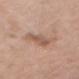This lesion was catalogued during total-body skin photography and was not selected for biopsy. Located on the front of the torso. A female subject roughly 40 years of age. A lesion tile, about 15 mm wide, cut from a 3D total-body photograph. Measured at roughly 3 mm in maximum diameter. Automated tile analysis of the lesion measured a footprint of about 5 mm² and two-axis asymmetry of about 0.25. The software also gave a lesion color around L≈55 a*≈19 b*≈28 in CIELAB, a lesion–skin lightness drop of about 9, and a normalized border contrast of about 6. The software also gave a classifier nevus-likeness of about 0/100 and a lesion-detection confidence of about 100/100.